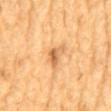workup — catalogued during a skin exam; not biopsied | patient — male, about 85 years old or older | location — the mid back | imaging modality — ~15 mm crop, total-body skin-cancer survey | image-analysis metrics — a classifier nevus-likeness of about 25/100 and a lesion-detection confidence of about 100/100 | illumination — cross-polarized | size — about 3.5 mm.